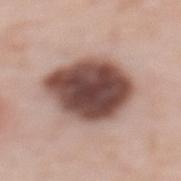The patient is a female aged 48 to 52. On the upper back. The recorded lesion diameter is about 8 mm. Automated image analysis of the tile measured a lesion area of about 34 mm², an eccentricity of roughly 0.7, and a shape-asymmetry score of about 0.15 (0 = symmetric). And it measured an average lesion color of about L≈47 a*≈19 b*≈22 (CIELAB) and a normalized border contrast of about 14.5. It also reported a classifier nevus-likeness of about 10/100 and lesion-presence confidence of about 100/100. This image is a 15 mm lesion crop taken from a total-body photograph.